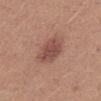No biopsy was performed on this lesion — it was imaged during a full skin examination and was not determined to be concerning. The patient is a male in their mid- to late 20s. Automated image analysis of the tile measured a mean CIELAB color near L≈49 a*≈22 b*≈24, a lesion–skin lightness drop of about 10, and a normalized border contrast of about 7.5. The analysis additionally found a border-irregularity index near 2/10. A roughly 15 mm field-of-view crop from a total-body skin photograph. Measured at roughly 4.5 mm in maximum diameter. On the abdomen.